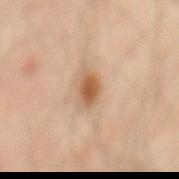The lesion is located on the mid back. A subject aged 53 to 57. Automated image analysis of the tile measured a lesion area of about 5.5 mm² and an eccentricity of roughly 0.75. The analysis additionally found border irregularity of about 2.5 on a 0–10 scale, internal color variation of about 3.5 on a 0–10 scale, and radial color variation of about 1. And it measured a classifier nevus-likeness of about 95/100. Approximately 3.5 mm at its widest. A 15 mm close-up extracted from a 3D total-body photography capture.